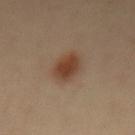The lesion was photographed on a routine skin check and not biopsied; there is no pathology result. The subject is a male aged around 40. About 4 mm across. A roughly 15 mm field-of-view crop from a total-body skin photograph. From the front of the torso.